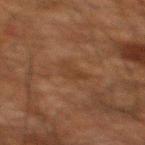Q: Is there a histopathology result?
A: total-body-photography surveillance lesion; no biopsy
Q: Patient demographics?
A: male, about 60 years old
Q: What is the anatomic site?
A: the mid back
Q: Lesion size?
A: ≈3 mm
Q: Automated lesion metrics?
A: a footprint of about 4.5 mm², an eccentricity of roughly 0.75, and a symmetry-axis asymmetry near 0.5
Q: How was this image acquired?
A: ~15 mm tile from a whole-body skin photo
Q: What lighting was used for the tile?
A: cross-polarized